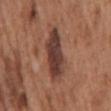No biopsy was performed on this lesion — it was imaged during a full skin examination and was not determined to be concerning. An algorithmic analysis of the crop reported an average lesion color of about L≈39 a*≈21 b*≈24 (CIELAB), about 13 CIELAB-L* units darker than the surrounding skin, and a normalized border contrast of about 11. And it measured a classifier nevus-likeness of about 30/100. The tile uses white-light illumination. From the mid back. This image is a 15 mm lesion crop taken from a total-body photograph. Longest diameter approximately 6 mm. The subject is a male aged approximately 65.